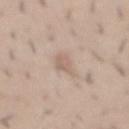No biopsy was performed on this lesion — it was imaged during a full skin examination and was not determined to be concerning.